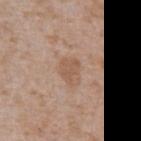Assessment:
The lesion was photographed on a routine skin check and not biopsied; there is no pathology result.
Image and clinical context:
About 3 mm across. Automated tile analysis of the lesion measured an area of roughly 5.5 mm², a shape eccentricity near 0.7, and a symmetry-axis asymmetry near 0.3. The software also gave a detector confidence of about 100 out of 100 that the crop contains a lesion. Cropped from a whole-body photographic skin survey; the tile spans about 15 mm. Captured under white-light illumination. A male subject, aged 63 to 67. Located on the abdomen.